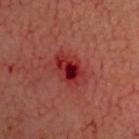workup: catalogued during a skin exam; not biopsied | imaging modality: 15 mm crop, total-body photography | diameter: about 4 mm | site: the head or neck | patient: in their mid-60s.